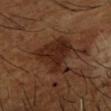No biopsy was performed on this lesion — it was imaged during a full skin examination and was not determined to be concerning. The lesion is located on the right forearm. The patient is a male aged approximately 65. A region of skin cropped from a whole-body photographic capture, roughly 15 mm wide.